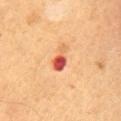Q: Was this lesion biopsied?
A: imaged on a skin check; not biopsied
Q: What are the patient's age and sex?
A: male, aged around 55
Q: What is the anatomic site?
A: the abdomen
Q: What is the imaging modality?
A: ~15 mm crop, total-body skin-cancer survey